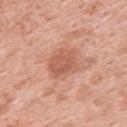Imaged during a routine full-body skin examination; the lesion was not biopsied and no histopathology is available. Automated tile analysis of the lesion measured a shape eccentricity near 0.55. And it measured a lesion color around L≈58 a*≈26 b*≈32 in CIELAB and a normalized lesion–skin contrast near 6.5. And it measured a nevus-likeness score of about 0/100 and lesion-presence confidence of about 100/100. A female patient, approximately 50 years of age. Captured under white-light illumination. From the upper back. Cropped from a whole-body photographic skin survey; the tile spans about 15 mm. Approximately 4 mm at its widest.